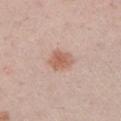Q: Was a biopsy performed?
A: imaged on a skin check; not biopsied
Q: What are the patient's age and sex?
A: male, aged around 50
Q: Where on the body is the lesion?
A: the left upper arm
Q: How was this image acquired?
A: 15 mm crop, total-body photography